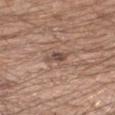location: the right forearm
image source: 15 mm crop, total-body photography
subject: male, aged around 20